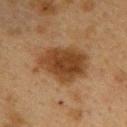workup: catalogued during a skin exam; not biopsied
patient: female, approximately 40 years of age
location: the upper back
image source: 15 mm crop, total-body photography